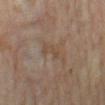The tile uses cross-polarized illumination. The lesion is on the left lower leg. The total-body-photography lesion software estimated radial color variation of about 0. The software also gave an automated nevus-likeness rating near 0 out of 100 and a lesion-detection confidence of about 95/100. A 15 mm crop from a total-body photograph taken for skin-cancer surveillance. The patient is a female aged 33–37.